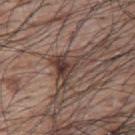<tbp_lesion>
  <biopsy_status>not biopsied; imaged during a skin examination</biopsy_status>
  <image>
    <source>total-body photography crop</source>
    <field_of_view_mm>15</field_of_view_mm>
  </image>
  <lighting>white-light</lighting>
  <lesion_size>
    <long_diameter_mm_approx>5.5</long_diameter_mm_approx>
  </lesion_size>
  <site>upper back</site>
  <patient>
    <sex>male</sex>
    <age_approx>70</age_approx>
  </patient>
</tbp_lesion>